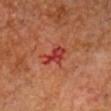{"biopsy_status": "not biopsied; imaged during a skin examination", "patient": {"sex": "male", "age_approx": 60}, "image": {"source": "total-body photography crop", "field_of_view_mm": 15}, "lesion_size": {"long_diameter_mm_approx": 3.0}, "site": "head or neck"}